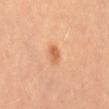workup: imaged on a skin check; not biopsied | subject: female, approximately 30 years of age | acquisition: total-body-photography crop, ~15 mm field of view | automated metrics: roughly 10 lightness units darker than nearby skin and a normalized lesion–skin contrast near 7; border irregularity of about 2.5 on a 0–10 scale, a color-variation rating of about 3.5/10, and radial color variation of about 1.5; lesion-presence confidence of about 100/100 | anatomic site: the right thigh | lesion size: about 2.5 mm | illumination: cross-polarized illumination.